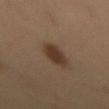Findings:
* subject: male, aged approximately 40
* image source: ~15 mm tile from a whole-body skin photo
* automated metrics: border irregularity of about 2.5 on a 0–10 scale, a color-variation rating of about 1/10, and a peripheral color-asymmetry measure near 0.5; a nevus-likeness score of about 100/100
* diameter: ≈2.5 mm
* body site: the lower back